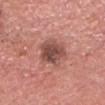Clinical impression:
The lesion was tiled from a total-body skin photograph and was not biopsied.
Image and clinical context:
From the head or neck. A roughly 15 mm field-of-view crop from a total-body skin photograph. An algorithmic analysis of the crop reported a border-irregularity rating of about 2.5/10 and a peripheral color-asymmetry measure near 1.5. The tile uses white-light illumination. A male patient, roughly 65 years of age.